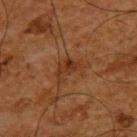– biopsy status — imaged on a skin check; not biopsied
– site — the upper back
– acquisition — total-body-photography crop, ~15 mm field of view
– patient — male, in their mid-60s